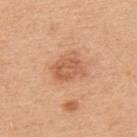The lesion was tiled from a total-body skin photograph and was not biopsied. About 3.5 mm across. From the upper back. A region of skin cropped from a whole-body photographic capture, roughly 15 mm wide. A male subject, roughly 50 years of age. The lesion-visualizer software estimated an average lesion color of about L≈59 a*≈25 b*≈36 (CIELAB), roughly 10 lightness units darker than nearby skin, and a normalized border contrast of about 6.5. The analysis additionally found a border-irregularity rating of about 2.5/10, a color-variation rating of about 2.5/10, and a peripheral color-asymmetry measure near 1. And it measured a nevus-likeness score of about 45/100 and lesion-presence confidence of about 100/100. This is a white-light tile.